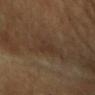<record>
  <biopsy_status>not biopsied; imaged during a skin examination</biopsy_status>
  <site>head or neck</site>
  <image>
    <source>total-body photography crop</source>
    <field_of_view_mm>15</field_of_view_mm>
  </image>
  <patient>
    <age_approx>60</age_approx>
  </patient>
</record>